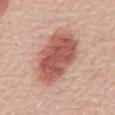The lesion was tiled from a total-body skin photograph and was not biopsied. The tile uses white-light illumination. A male subject, aged around 70. Longest diameter approximately 7 mm. Cropped from a total-body skin-imaging series; the visible field is about 15 mm. On the mid back.